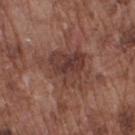follow-up = total-body-photography surveillance lesion; no biopsy | TBP lesion metrics = an average lesion color of about L≈39 a*≈20 b*≈23 (CIELAB), roughly 9 lightness units darker than nearby skin, and a normalized lesion–skin contrast near 7.5; an automated nevus-likeness rating near 5 out of 100 and a detector confidence of about 100 out of 100 that the crop contains a lesion | illumination = white-light illumination | size = ≈6 mm | anatomic site = the right upper arm | image source = total-body-photography crop, ~15 mm field of view | subject = male, aged 73 to 77.